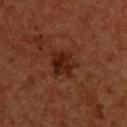Part of a total-body skin-imaging series; this lesion was reviewed on a skin check and was not flagged for biopsy. The total-body-photography lesion software estimated a border-irregularity rating of about 2.5/10, internal color variation of about 4 on a 0–10 scale, and peripheral color asymmetry of about 1.5. The software also gave a classifier nevus-likeness of about 85/100. Located on the upper back. The recorded lesion diameter is about 3.5 mm. Imaged with cross-polarized lighting. The subject is a male aged approximately 55. A region of skin cropped from a whole-body photographic capture, roughly 15 mm wide.